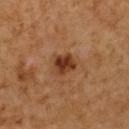Q: Patient demographics?
A: male, in their 60s
Q: Illumination type?
A: cross-polarized illumination
Q: What kind of image is this?
A: 15 mm crop, total-body photography
Q: Automated lesion metrics?
A: an average lesion color of about L≈33 a*≈22 b*≈32 (CIELAB), about 12 CIELAB-L* units darker than the surrounding skin, and a lesion-to-skin contrast of about 10.5 (normalized; higher = more distinct); an automated nevus-likeness rating near 95 out of 100 and a detector confidence of about 100 out of 100 that the crop contains a lesion
Q: How large is the lesion?
A: ≈3 mm
Q: Where on the body is the lesion?
A: the upper back